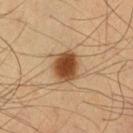Assessment:
Part of a total-body skin-imaging series; this lesion was reviewed on a skin check and was not flagged for biopsy.
Clinical summary:
About 4 mm across. A region of skin cropped from a whole-body photographic capture, roughly 15 mm wide. The lesion is on the chest. The subject is a male aged 53–57. The tile uses cross-polarized illumination.